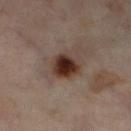Notes:
– follow-up · total-body-photography surveillance lesion; no biopsy
– location · the left lower leg
– patient · female, approximately 55 years of age
– acquisition · total-body-photography crop, ~15 mm field of view